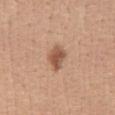Image and clinical context:
A female patient, aged around 40. A close-up tile cropped from a whole-body skin photograph, about 15 mm across. Located on the abdomen. Longest diameter approximately 3 mm.